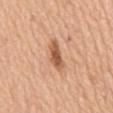<case>
<biopsy_status>not biopsied; imaged during a skin examination</biopsy_status>
<patient>
  <sex>female</sex>
  <age_approx>55</age_approx>
</patient>
<lesion_size>
  <long_diameter_mm_approx>4.0</long_diameter_mm_approx>
</lesion_size>
<site>mid back</site>
<lighting>white-light</lighting>
<image>
  <source>total-body photography crop</source>
  <field_of_view_mm>15</field_of_view_mm>
</image>
</case>